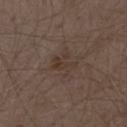Captured during whole-body skin photography for melanoma surveillance; the lesion was not biopsied.
A male subject, approximately 70 years of age.
The recorded lesion diameter is about 3 mm.
From the abdomen.
This image is a 15 mm lesion crop taken from a total-body photograph.
The lesion-visualizer software estimated a lesion area of about 6 mm² and a symmetry-axis asymmetry near 0.5. The analysis additionally found a lesion color around L≈35 a*≈13 b*≈22 in CIELAB and a normalized lesion–skin contrast near 5.5. And it measured a border-irregularity index near 6/10 and a within-lesion color-variation index near 3/10. The software also gave an automated nevus-likeness rating near 0 out of 100 and a lesion-detection confidence of about 100/100.
The tile uses white-light illumination.